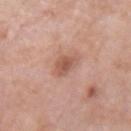<case>
<biopsy_status>not biopsied; imaged during a skin examination</biopsy_status>
<lesion_size>
  <long_diameter_mm_approx>2.5</long_diameter_mm_approx>
</lesion_size>
<lighting>white-light</lighting>
<site>right forearm</site>
<image>
  <source>total-body photography crop</source>
  <field_of_view_mm>15</field_of_view_mm>
</image>
<patient>
  <sex>female</sex>
  <age_approx>70</age_approx>
</patient>
</case>